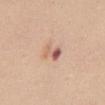biopsy_status: not biopsied; imaged during a skin examination
lighting: white-light
image:
  source: total-body photography crop
  field_of_view_mm: 15
patient:
  sex: female
  age_approx: 30
site: back